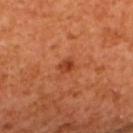A 15 mm close-up extracted from a 3D total-body photography capture. From the upper back. The lesion's longest dimension is about 2 mm. The tile uses cross-polarized illumination. A female patient, in their mid-50s.